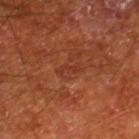This lesion was catalogued during total-body skin photography and was not selected for biopsy.
The subject is a male aged 63–67.
Automated tile analysis of the lesion measured a footprint of about 2 mm², a shape eccentricity near 0.9, and a shape-asymmetry score of about 0.55 (0 = symmetric). The software also gave border irregularity of about 6.5 on a 0–10 scale, a within-lesion color-variation index near 0/10, and radial color variation of about 0.
Approximately 2.5 mm at its widest.
On the right lower leg.
This is a cross-polarized tile.
Cropped from a total-body skin-imaging series; the visible field is about 15 mm.